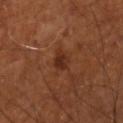A male patient, aged around 70. The tile uses cross-polarized illumination. A lesion tile, about 15 mm wide, cut from a 3D total-body photograph. Located on the right lower leg. Measured at roughly 2.5 mm in maximum diameter.